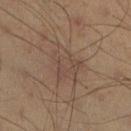Captured during whole-body skin photography for melanoma surveillance; the lesion was not biopsied. A male patient, aged 38 to 42. The lesion is located on the right lower leg. A close-up tile cropped from a whole-body skin photograph, about 15 mm across.